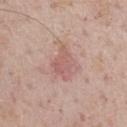biopsy status=catalogued during a skin exam; not biopsied | patient=male, aged approximately 75 | body site=the front of the torso | image=~15 mm tile from a whole-body skin photo.